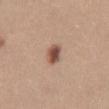Case summary:
• workup · catalogued during a skin exam; not biopsied
• subject · female, about 25 years old
• automated lesion analysis · border irregularity of about 1 on a 0–10 scale, internal color variation of about 4.5 on a 0–10 scale, and peripheral color asymmetry of about 1.5
• body site · the front of the torso
• lesion size · ~2.5 mm (longest diameter)
• acquisition · ~15 mm tile from a whole-body skin photo
• tile lighting · white-light illumination